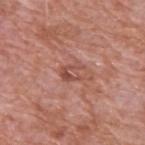Q: Was this lesion biopsied?
A: imaged on a skin check; not biopsied
Q: What is the imaging modality?
A: ~15 mm tile from a whole-body skin photo
Q: Lesion location?
A: the upper back
Q: What are the patient's age and sex?
A: male, roughly 60 years of age